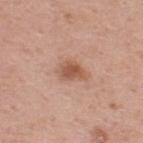| field | value |
|---|---|
| biopsy status | total-body-photography surveillance lesion; no biopsy |
| automated metrics | a mean CIELAB color near L≈54 a*≈23 b*≈30, a lesion–skin lightness drop of about 11, and a lesion-to-skin contrast of about 8 (normalized; higher = more distinct); border irregularity of about 2.5 on a 0–10 scale, a within-lesion color-variation index near 2.5/10, and a peripheral color-asymmetry measure near 1 |
| image source | ~15 mm crop, total-body skin-cancer survey |
| diameter | ~3 mm (longest diameter) |
| lighting | white-light illumination |
| location | the upper back |
| patient | male, about 40 years old |